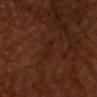The lesion was tiled from a total-body skin photograph and was not biopsied. A male patient aged approximately 65. Located on the chest. A 15 mm crop from a total-body photograph taken for skin-cancer surveillance.